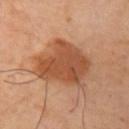| field | value |
|---|---|
| workup | imaged on a skin check; not biopsied |
| body site | the left upper arm |
| image source | total-body-photography crop, ~15 mm field of view |
| illumination | cross-polarized illumination |
| subject | female, about 45 years old |
| lesion diameter | ≈6 mm |